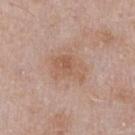Assessment:
Captured during whole-body skin photography for melanoma surveillance; the lesion was not biopsied.
Image and clinical context:
A 15 mm crop from a total-body photograph taken for skin-cancer surveillance. An algorithmic analysis of the crop reported a mean CIELAB color near L≈57 a*≈19 b*≈30, roughly 7 lightness units darker than nearby skin, and a lesion-to-skin contrast of about 5.5 (normalized; higher = more distinct). A male subject in their mid-50s. The lesion's longest dimension is about 4 mm. The lesion is located on the chest.